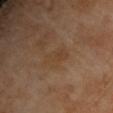The lesion was tiled from a total-body skin photograph and was not biopsied. This image is a 15 mm lesion crop taken from a total-body photograph. The lesion is located on the chest. The recorded lesion diameter is about 3.5 mm. Automated tile analysis of the lesion measured an area of roughly 4.5 mm², a shape eccentricity near 0.85, and two-axis asymmetry of about 0.3. And it measured a lesion color around L≈39 a*≈16 b*≈30 in CIELAB, roughly 4 lightness units darker than nearby skin, and a normalized lesion–skin contrast near 5. And it measured border irregularity of about 3.5 on a 0–10 scale and internal color variation of about 1.5 on a 0–10 scale. The patient is a male aged around 70. The tile uses cross-polarized illumination.